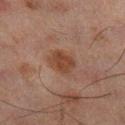No biopsy was performed on this lesion — it was imaged during a full skin examination and was not determined to be concerning. The recorded lesion diameter is about 3.5 mm. The subject is a male in their mid- to late 40s. Imaged with cross-polarized lighting. The lesion is on the right lower leg. A 15 mm crop from a total-body photograph taken for skin-cancer surveillance.